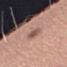Assessment: No biopsy was performed on this lesion — it was imaged during a full skin examination and was not determined to be concerning. Image and clinical context: The lesion is on the arm. This is a white-light tile. The patient is a female aged approximately 40. A region of skin cropped from a whole-body photographic capture, roughly 15 mm wide. Measured at roughly 3 mm in maximum diameter.